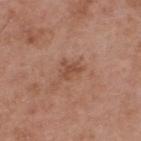Assessment:
Part of a total-body skin-imaging series; this lesion was reviewed on a skin check and was not flagged for biopsy.
Acquisition and patient details:
A region of skin cropped from a whole-body photographic capture, roughly 15 mm wide. A male patient approximately 55 years of age. Automated image analysis of the tile measured an average lesion color of about L≈49 a*≈23 b*≈30 (CIELAB), a lesion–skin lightness drop of about 8, and a normalized lesion–skin contrast near 6. The software also gave border irregularity of about 5.5 on a 0–10 scale and peripheral color asymmetry of about 0.5. This is a white-light tile. The lesion is on the upper back. The lesion's longest dimension is about 2.5 mm.